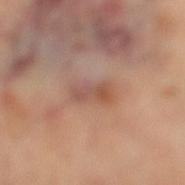No biopsy was performed on this lesion — it was imaged during a full skin examination and was not determined to be concerning.
A female patient, aged around 60.
The tile uses cross-polarized illumination.
Located on the right leg.
Cropped from a total-body skin-imaging series; the visible field is about 15 mm.
Measured at roughly 4 mm in maximum diameter.
An algorithmic analysis of the crop reported a shape-asymmetry score of about 0.25 (0 = symmetric). And it measured a lesion color around L≈53 a*≈21 b*≈28 in CIELAB, about 8 CIELAB-L* units darker than the surrounding skin, and a normalized border contrast of about 5.5. And it measured border irregularity of about 3.5 on a 0–10 scale and peripheral color asymmetry of about 2. It also reported a classifier nevus-likeness of about 0/100 and a detector confidence of about 100 out of 100 that the crop contains a lesion.